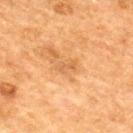Clinical impression:
No biopsy was performed on this lesion — it was imaged during a full skin examination and was not determined to be concerning.
Acquisition and patient details:
A male subject, aged 48–52. The lesion is located on the back. A lesion tile, about 15 mm wide, cut from a 3D total-body photograph.